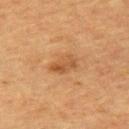{"biopsy_status": "not biopsied; imaged during a skin examination", "image": {"source": "total-body photography crop", "field_of_view_mm": 15}, "lesion_size": {"long_diameter_mm_approx": 3.5}, "site": "left upper arm", "automated_metrics": {"cielab_L": 43, "cielab_a": 19, "cielab_b": 33, "vs_skin_darker_L": 7.0}, "lighting": "cross-polarized", "patient": {"sex": "female", "age_approx": 55}}